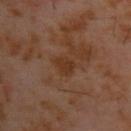The lesion was photographed on a routine skin check and not biopsied; there is no pathology result.
Cropped from a whole-body photographic skin survey; the tile spans about 15 mm.
The lesion is on the back.
A male subject, aged approximately 60.